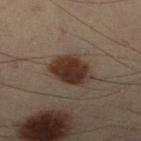Assessment:
No biopsy was performed on this lesion — it was imaged during a full skin examination and was not determined to be concerning.
Acquisition and patient details:
A 15 mm crop from a total-body photograph taken for skin-cancer surveillance. Approximately 4 mm at its widest. The lesion-visualizer software estimated a border-irregularity index near 1.5/10 and peripheral color asymmetry of about 1. It also reported a nevus-likeness score of about 95/100 and a lesion-detection confidence of about 100/100. On the right lower leg. A male subject about 55 years old. This is a cross-polarized tile.